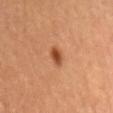Clinical impression:
Part of a total-body skin-imaging series; this lesion was reviewed on a skin check and was not flagged for biopsy.
Context:
A male subject roughly 60 years of age. The lesion is on the mid back. A 15 mm crop from a total-body photograph taken for skin-cancer surveillance.